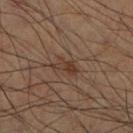| field | value |
|---|---|
| follow-up | imaged on a skin check; not biopsied |
| site | the right lower leg |
| subject | male, roughly 60 years of age |
| imaging modality | total-body-photography crop, ~15 mm field of view |
| lesion size | ~3.5 mm (longest diameter) |